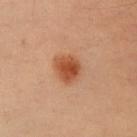Assessment:
Imaged during a routine full-body skin examination; the lesion was not biopsied and no histopathology is available.
Background:
Measured at roughly 3.5 mm in maximum diameter. A male subject, aged approximately 55. A 15 mm close-up extracted from a 3D total-body photography capture. Located on the right upper arm.